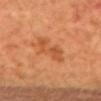follow-up = no biopsy performed (imaged during a skin exam)
location = the back
diameter = ~4.5 mm (longest diameter)
imaging modality = total-body-photography crop, ~15 mm field of view
tile lighting = cross-polarized
subject = female, aged approximately 40